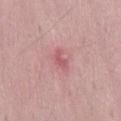No biopsy was performed on this lesion — it was imaged during a full skin examination and was not determined to be concerning.
A 15 mm close-up tile from a total-body photography series done for melanoma screening.
The lesion is located on the mid back.
A male subject about 50 years old.
Measured at roughly 2.5 mm in maximum diameter.
The tile uses white-light illumination.